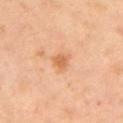No biopsy was performed on this lesion — it was imaged during a full skin examination and was not determined to be concerning.